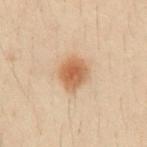Assessment:
The lesion was photographed on a routine skin check and not biopsied; there is no pathology result.
Acquisition and patient details:
A 15 mm close-up extracted from a 3D total-body photography capture. Approximately 3.5 mm at its widest. The lesion is on the abdomen. A male subject, aged 28–32. Imaged with cross-polarized lighting.